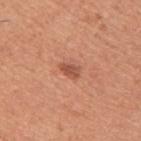biopsy status: imaged on a skin check; not biopsied | site: the upper back | image: ~15 mm crop, total-body skin-cancer survey | subject: male, in their mid-40s.